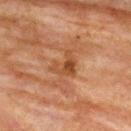This lesion was catalogued during total-body skin photography and was not selected for biopsy.
A roughly 15 mm field-of-view crop from a total-body skin photograph.
Approximately 3 mm at its widest.
Captured under cross-polarized illumination.
A female subject aged around 80.
The lesion is on the back.